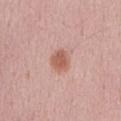Recorded during total-body skin imaging; not selected for excision or biopsy. An algorithmic analysis of the crop reported a peripheral color-asymmetry measure near 0.5. A lesion tile, about 15 mm wide, cut from a 3D total-body photograph. A male patient, aged 58–62. The tile uses white-light illumination. Longest diameter approximately 3 mm. On the abdomen.